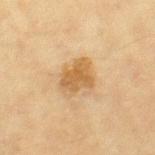Clinical impression: The lesion was tiled from a total-body skin photograph and was not biopsied. Background: A lesion tile, about 15 mm wide, cut from a 3D total-body photograph. A female patient, in their mid-50s. On the right thigh.